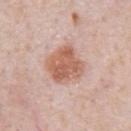| field | value |
|---|---|
| follow-up | total-body-photography surveillance lesion; no biopsy |
| subject | male, in their 80s |
| acquisition | 15 mm crop, total-body photography |
| body site | the chest |
| size | ≈4.5 mm |